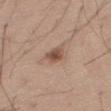Part of a total-body skin-imaging series; this lesion was reviewed on a skin check and was not flagged for biopsy. The patient is a male in their mid-30s. The lesion is on the right thigh. A 15 mm close-up extracted from a 3D total-body photography capture. An algorithmic analysis of the crop reported a lesion area of about 4.5 mm², an outline eccentricity of about 0.65 (0 = round, 1 = elongated), and a symmetry-axis asymmetry near 0.25. It also reported about 12 CIELAB-L* units darker than the surrounding skin and a normalized border contrast of about 8. This is a white-light tile. Measured at roughly 2.5 mm in maximum diameter.